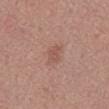{
  "biopsy_status": "not biopsied; imaged during a skin examination",
  "image": {
    "source": "total-body photography crop",
    "field_of_view_mm": 15
  },
  "patient": {
    "sex": "male",
    "age_approx": 55
  },
  "lesion_size": {
    "long_diameter_mm_approx": 3.0
  },
  "automated_metrics": {
    "area_mm2_approx": 4.5,
    "eccentricity": 0.8,
    "cielab_L": 53,
    "cielab_a": 22,
    "cielab_b": 25,
    "vs_skin_darker_L": 7.0,
    "border_irregularity_0_10": 3.5,
    "color_variation_0_10": 1.5,
    "peripheral_color_asymmetry": 0.5,
    "nevus_likeness_0_100": 5,
    "lesion_detection_confidence_0_100": 100
  },
  "site": "mid back",
  "lighting": "white-light"
}